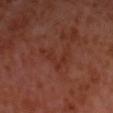Findings:
* workup · total-body-photography surveillance lesion; no biopsy
* TBP lesion metrics · a border-irregularity index near 9.5/10, internal color variation of about 1 on a 0–10 scale, and radial color variation of about 0.5
* location · the head or neck
* tile lighting · cross-polarized illumination
* subject · male, aged 53–57
* diameter · about 4.5 mm
* imaging modality · total-body-photography crop, ~15 mm field of view